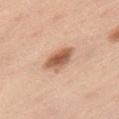| key | value |
|---|---|
| workup | no biopsy performed (imaged during a skin exam) |
| acquisition | ~15 mm tile from a whole-body skin photo |
| patient | male, about 50 years old |
| body site | the lower back |
| automated lesion analysis | a border-irregularity rating of about 2.5/10, a color-variation rating of about 4.5/10, and a peripheral color-asymmetry measure near 1.5 |
| lighting | white-light illumination |
| lesion size | about 4 mm |